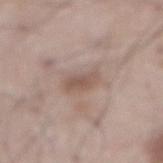Background:
From the abdomen. A 15 mm close-up extracted from a 3D total-body photography capture. A male patient approximately 55 years of age.